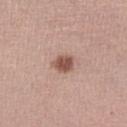follow-up: total-body-photography surveillance lesion; no biopsy | acquisition: total-body-photography crop, ~15 mm field of view | site: the right lower leg | lighting: white-light | diameter: ~3 mm (longest diameter) | patient: female, aged approximately 60.